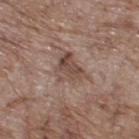A male subject, aged 68–72. A 15 mm close-up tile from a total-body photography series done for melanoma screening. The recorded lesion diameter is about 4 mm. The lesion-visualizer software estimated a footprint of about 8.5 mm², an outline eccentricity of about 0.7 (0 = round, 1 = elongated), and a symmetry-axis asymmetry near 0.4. The software also gave an average lesion color of about L≈47 a*≈17 b*≈23 (CIELAB), a lesion–skin lightness drop of about 9, and a lesion-to-skin contrast of about 7 (normalized; higher = more distinct). On the upper back.